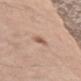The lesion was photographed on a routine skin check and not biopsied; there is no pathology result.
Located on the left upper arm.
Measured at roughly 2.5 mm in maximum diameter.
The subject is a male aged 68 to 72.
The total-body-photography lesion software estimated a footprint of about 3 mm², an outline eccentricity of about 0.85 (0 = round, 1 = elongated), and a shape-asymmetry score of about 0.3 (0 = symmetric). And it measured a lesion color around L≈58 a*≈19 b*≈28 in CIELAB, a lesion–skin lightness drop of about 10, and a normalized border contrast of about 6.5.
A 15 mm close-up extracted from a 3D total-body photography capture.